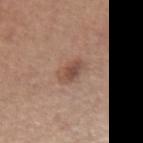Q: What lighting was used for the tile?
A: white-light illumination
Q: What is the lesion's diameter?
A: about 4 mm
Q: What are the patient's age and sex?
A: female, approximately 65 years of age
Q: What is the imaging modality?
A: ~15 mm tile from a whole-body skin photo
Q: Lesion location?
A: the right forearm